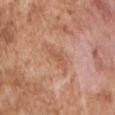The lesion is located on the chest. A male subject aged 63–67. The total-body-photography lesion software estimated an area of roughly 4.5 mm² and a symmetry-axis asymmetry near 0.35. It also reported an average lesion color of about L≈57 a*≈23 b*≈33 (CIELAB). Captured under white-light illumination. A region of skin cropped from a whole-body photographic capture, roughly 15 mm wide. The recorded lesion diameter is about 4 mm.